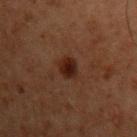This lesion was catalogued during total-body skin photography and was not selected for biopsy.
Located on the left upper arm.
A male patient, aged 58 to 62.
About 2.5 mm across.
The lesion-visualizer software estimated a border-irregularity rating of about 2.5/10, internal color variation of about 3 on a 0–10 scale, and a peripheral color-asymmetry measure near 1. The analysis additionally found a nevus-likeness score of about 95/100 and a detector confidence of about 100 out of 100 that the crop contains a lesion.
Imaged with cross-polarized lighting.
A roughly 15 mm field-of-view crop from a total-body skin photograph.